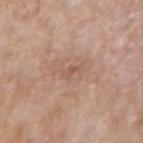biopsy status: total-body-photography surveillance lesion; no biopsy
patient: female, aged around 70
acquisition: ~15 mm crop, total-body skin-cancer survey
TBP lesion metrics: a lesion area of about 2.5 mm², an outline eccentricity of about 0.9 (0 = round, 1 = elongated), and a shape-asymmetry score of about 0.35 (0 = symmetric); an average lesion color of about L≈56 a*≈20 b*≈29 (CIELAB) and roughly 7 lightness units darker than nearby skin
body site: the left forearm
illumination: white-light
size: about 2.5 mm